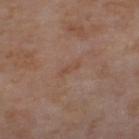Longest diameter approximately 2.5 mm.
On the left thigh.
This image is a 15 mm lesion crop taken from a total-body photograph.
The subject is a female approximately 55 years of age.
Captured under cross-polarized illumination.